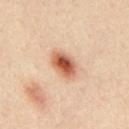Captured during whole-body skin photography for melanoma surveillance; the lesion was not biopsied. The lesion is on the back. A male subject, in their mid- to late 30s. A region of skin cropped from a whole-body photographic capture, roughly 15 mm wide.